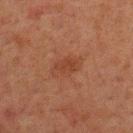image source — ~15 mm crop, total-body skin-cancer survey | site — the upper back | patient — male, aged around 60 | lesion diameter — about 4 mm.